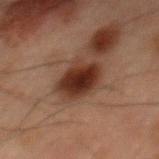This lesion was catalogued during total-body skin photography and was not selected for biopsy. An algorithmic analysis of the crop reported an area of roughly 13 mm², an eccentricity of roughly 0.75, and two-axis asymmetry of about 0.15. This is a cross-polarized tile. A roughly 15 mm field-of-view crop from a total-body skin photograph. The patient is a male aged approximately 60. The lesion is located on the mid back.